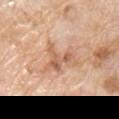No biopsy was performed on this lesion — it was imaged during a full skin examination and was not determined to be concerning.
A male patient aged around 60.
From the chest.
Longest diameter approximately 4 mm.
Captured under white-light illumination.
This image is a 15 mm lesion crop taken from a total-body photograph.